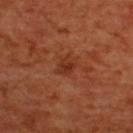Recorded during total-body skin imaging; not selected for excision or biopsy. Cropped from a whole-body photographic skin survey; the tile spans about 15 mm. Longest diameter approximately 2.5 mm. Automated tile analysis of the lesion measured an outline eccentricity of about 0.6 (0 = round, 1 = elongated) and two-axis asymmetry of about 0.3. And it measured a lesion color around L≈35 a*≈29 b*≈35 in CIELAB, a lesion–skin lightness drop of about 7, and a normalized lesion–skin contrast near 6.5. It also reported a within-lesion color-variation index near 2.5/10 and peripheral color asymmetry of about 1. The lesion is on the upper back. Captured under cross-polarized illumination. A female patient aged 53–57.